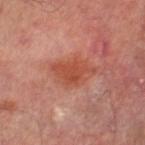Notes:
- workup — total-body-photography surveillance lesion; no biopsy
- anatomic site — the right thigh
- lighting — cross-polarized illumination
- image — ~15 mm tile from a whole-body skin photo
- automated metrics — a lesion color around L≈47 a*≈29 b*≈32 in CIELAB, roughly 8 lightness units darker than nearby skin, and a normalized border contrast of about 7.5; a border-irregularity index near 5/10 and radial color variation of about 0.5; a classifier nevus-likeness of about 0/100 and a lesion-detection confidence of about 100/100
- subject — male, in their mid-60s
- lesion size — ≈4.5 mm